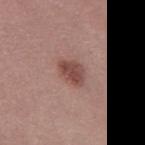follow-up: imaged on a skin check; not biopsied
site: the left thigh
lighting: white-light illumination
diameter: about 3.5 mm
imaging modality: ~15 mm tile from a whole-body skin photo
subject: female, approximately 50 years of age